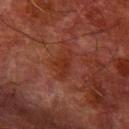Notes:
- workup — catalogued during a skin exam; not biopsied
- site — the right forearm
- tile lighting — cross-polarized
- size — ~3.5 mm (longest diameter)
- imaging modality — 15 mm crop, total-body photography
- automated metrics — a footprint of about 5 mm², an outline eccentricity of about 0.85 (0 = round, 1 = elongated), and a shape-asymmetry score of about 0.35 (0 = symmetric); an automated nevus-likeness rating near 0 out of 100
- subject — male, aged approximately 80